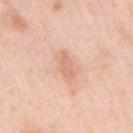Image and clinical context: This is a white-light tile. Measured at roughly 2.5 mm in maximum diameter. A roughly 15 mm field-of-view crop from a total-body skin photograph. The lesion is on the right upper arm. A female subject aged 63 to 67.